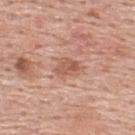follow-up: imaged on a skin check; not biopsied | imaging modality: 15 mm crop, total-body photography | automated metrics: a lesion color around L≈57 a*≈23 b*≈30 in CIELAB; border irregularity of about 3.5 on a 0–10 scale, a color-variation rating of about 3.5/10, and peripheral color asymmetry of about 1 | patient: male, about 55 years old | location: the upper back.